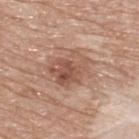No biopsy was performed on this lesion — it was imaged during a full skin examination and was not determined to be concerning.
The subject is a male aged 73–77.
Captured under white-light illumination.
About 5 mm across.
A 15 mm crop from a total-body photograph taken for skin-cancer surveillance.
The lesion is on the upper back.